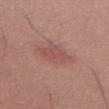This lesion was catalogued during total-body skin photography and was not selected for biopsy. A female patient, aged approximately 65. From the leg. About 5 mm across. A close-up tile cropped from a whole-body skin photograph, about 15 mm across.